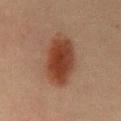Image and clinical context:
About 7.5 mm across. From the chest. Cropped from a whole-body photographic skin survey; the tile spans about 15 mm. A male patient about 60 years old. This is a cross-polarized tile. An algorithmic analysis of the crop reported a classifier nevus-likeness of about 100/100 and a detector confidence of about 100 out of 100 that the crop contains a lesion.